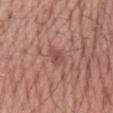Imaged during a routine full-body skin examination; the lesion was not biopsied and no histopathology is available. Automated tile analysis of the lesion measured a footprint of about 3.5 mm² and a shape eccentricity near 0.75. The software also gave a border-irregularity rating of about 2/10. On the right forearm. A roughly 15 mm field-of-view crop from a total-body skin photograph. This is a white-light tile. A male patient, aged around 50. Measured at roughly 2.5 mm in maximum diameter.